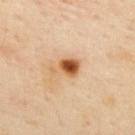Q: Was this lesion biopsied?
A: imaged on a skin check; not biopsied
Q: What kind of image is this?
A: ~15 mm tile from a whole-body skin photo
Q: Who is the patient?
A: female, approximately 40 years of age
Q: Lesion location?
A: the upper back